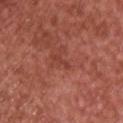Case summary:
• acquisition — 15 mm crop, total-body photography
• subject — male, approximately 65 years of age
• site — the chest
• lighting — white-light